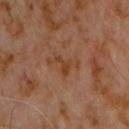Case summary:
- subject · male, aged 58–62
- site · the chest
- imaging modality · 15 mm crop, total-body photography